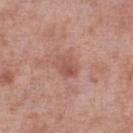Q: Was a biopsy performed?
A: catalogued during a skin exam; not biopsied
Q: How was the tile lit?
A: white-light illumination
Q: What is the imaging modality?
A: 15 mm crop, total-body photography
Q: Patient demographics?
A: male, in their mid- to late 50s
Q: Lesion size?
A: about 3.5 mm
Q: Where on the body is the lesion?
A: the left lower leg
Q: Automated lesion metrics?
A: a footprint of about 5.5 mm², a shape eccentricity near 0.75, and a symmetry-axis asymmetry near 0.2; an average lesion color of about L≈53 a*≈24 b*≈26 (CIELAB) and a normalized lesion–skin contrast near 5.5; a color-variation rating of about 4/10 and radial color variation of about 1.5